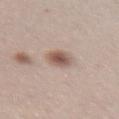Recorded during total-body skin imaging; not selected for excision or biopsy.
About 3.5 mm across.
A close-up tile cropped from a whole-body skin photograph, about 15 mm across.
Captured under white-light illumination.
On the front of the torso.
The subject is a female roughly 30 years of age.